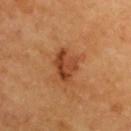Recorded during total-body skin imaging; not selected for excision or biopsy. About 4 mm across. Imaged with cross-polarized lighting. A close-up tile cropped from a whole-body skin photograph, about 15 mm across. A subject in their 70s. Located on the upper back. An algorithmic analysis of the crop reported a footprint of about 8.5 mm², an eccentricity of roughly 0.6, and a shape-asymmetry score of about 0.25 (0 = symmetric). The software also gave a lesion color around L≈43 a*≈27 b*≈37 in CIELAB and about 10 CIELAB-L* units darker than the surrounding skin. It also reported border irregularity of about 3 on a 0–10 scale, a color-variation rating of about 5.5/10, and a peripheral color-asymmetry measure near 2.